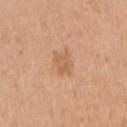Part of a total-body skin-imaging series; this lesion was reviewed on a skin check and was not flagged for biopsy.
On the right upper arm.
The subject is a female about 45 years old.
Imaged with white-light lighting.
A 15 mm close-up extracted from a 3D total-body photography capture.
Approximately 2.5 mm at its widest.